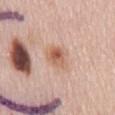A 15 mm close-up extracted from a 3D total-body photography capture. Imaged with white-light lighting. Located on the mid back. The lesion's longest dimension is about 4 mm. A female subject, in their mid-60s. An algorithmic analysis of the crop reported an automated nevus-likeness rating near 80 out of 100.